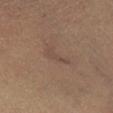No biopsy was performed on this lesion — it was imaged during a full skin examination and was not determined to be concerning.
Located on the right lower leg.
A 15 mm close-up extracted from a 3D total-body photography capture.
About 3.5 mm across.
Automated tile analysis of the lesion measured a lesion area of about 4 mm², a shape eccentricity near 0.9, and a shape-asymmetry score of about 0.5 (0 = symmetric). The software also gave border irregularity of about 6 on a 0–10 scale, internal color variation of about 0.5 on a 0–10 scale, and peripheral color asymmetry of about 0. The analysis additionally found a classifier nevus-likeness of about 0/100 and a lesion-detection confidence of about 75/100.
Imaged with cross-polarized lighting.
The subject is a female aged 28–32.